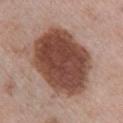{"biopsy_status": "not biopsied; imaged during a skin examination", "automated_metrics": {"eccentricity": 0.65, "shape_asymmetry": 0.15, "cielab_L": 46, "cielab_a": 21, "cielab_b": 26, "border_irregularity_0_10": 2.0, "color_variation_0_10": 5.0, "peripheral_color_asymmetry": 1.5, "nevus_likeness_0_100": 95}, "patient": {"sex": "female", "age_approx": 65}, "site": "right upper arm", "lighting": "white-light", "image": {"source": "total-body photography crop", "field_of_view_mm": 15}}